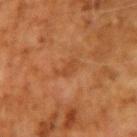follow-up — imaged on a skin check; not biopsied
image source — total-body-photography crop, ~15 mm field of view
subject — male, aged 68–72
body site — the arm